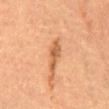The lesion is located on the abdomen.
The lesion's longest dimension is about 4 mm.
A female subject, about 70 years old.
The total-body-photography lesion software estimated a lesion area of about 4.5 mm², an outline eccentricity of about 0.95 (0 = round, 1 = elongated), and two-axis asymmetry of about 0.35. It also reported a lesion color around L≈50 a*≈21 b*≈34 in CIELAB, about 10 CIELAB-L* units darker than the surrounding skin, and a normalized border contrast of about 7.5. The software also gave a within-lesion color-variation index near 2/10 and radial color variation of about 0.5. It also reported an automated nevus-likeness rating near 10 out of 100 and a detector confidence of about 100 out of 100 that the crop contains a lesion.
Cropped from a whole-body photographic skin survey; the tile spans about 15 mm.